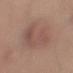{"biopsy_status": "not biopsied; imaged during a skin examination", "patient": {"sex": "male", "age_approx": 50}, "lesion_size": {"long_diameter_mm_approx": 7.0}, "lighting": "white-light", "site": "arm", "automated_metrics": {"eccentricity": 0.7}, "image": {"source": "total-body photography crop", "field_of_view_mm": 15}}